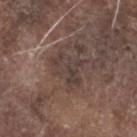This lesion was catalogued during total-body skin photography and was not selected for biopsy. Longest diameter approximately 4.5 mm. Automated tile analysis of the lesion measured an outline eccentricity of about 0.5 (0 = round, 1 = elongated) and a symmetry-axis asymmetry near 0.5. The analysis additionally found an average lesion color of about L≈38 a*≈13 b*≈18 (CIELAB) and roughly 7 lightness units darker than nearby skin. The lesion is located on the head or neck. Cropped from a whole-body photographic skin survey; the tile spans about 15 mm. Captured under white-light illumination. The patient is a male roughly 55 years of age.